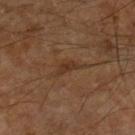{
  "biopsy_status": "not biopsied; imaged during a skin examination",
  "lesion_size": {
    "long_diameter_mm_approx": 3.0
  },
  "image": {
    "source": "total-body photography crop",
    "field_of_view_mm": 15
  },
  "patient": {
    "sex": "male",
    "age_approx": 60
  },
  "lighting": "cross-polarized",
  "site": "right lower leg"
}